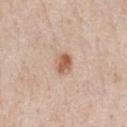Q: Was a biopsy performed?
A: total-body-photography surveillance lesion; no biopsy
Q: How large is the lesion?
A: about 2.5 mm
Q: Patient demographics?
A: male, about 60 years old
Q: What is the anatomic site?
A: the chest
Q: Illumination type?
A: white-light illumination
Q: How was this image acquired?
A: ~15 mm crop, total-body skin-cancer survey
Q: Automated lesion metrics?
A: an average lesion color of about L≈60 a*≈21 b*≈32 (CIELAB), a lesion–skin lightness drop of about 12, and a normalized lesion–skin contrast near 8.5; a border-irregularity index near 1.5/10, a within-lesion color-variation index near 5/10, and radial color variation of about 1.5; a classifier nevus-likeness of about 95/100